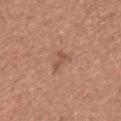Case summary:
* follow-up · total-body-photography surveillance lesion; no biopsy
* lesion size · ~2.5 mm (longest diameter)
* site · the front of the torso
* image source · ~15 mm tile from a whole-body skin photo
* patient · male, about 45 years old
* image-analysis metrics · border irregularity of about 4.5 on a 0–10 scale, a within-lesion color-variation index near 0.5/10, and radial color variation of about 0; a classifier nevus-likeness of about 0/100 and a lesion-detection confidence of about 100/100
* illumination · white-light